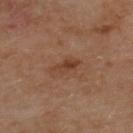<lesion>
<site>upper back</site>
<patient>
  <sex>female</sex>
  <age_approx>60</age_approx>
</patient>
<image>
  <source>total-body photography crop</source>
  <field_of_view_mm>15</field_of_view_mm>
</image>
<lesion_size>
  <long_diameter_mm_approx>4.0</long_diameter_mm_approx>
</lesion_size>
<automated_metrics>
  <border_irregularity_0_10>3.5</border_irregularity_0_10>
  <color_variation_0_10>4.0</color_variation_0_10>
  <peripheral_color_asymmetry>1.5</peripheral_color_asymmetry>
  <nevus_likeness_0_100>20</nevus_likeness_0_100>
  <lesion_detection_confidence_0_100>100</lesion_detection_confidence_0_100>
</automated_metrics>
</lesion>